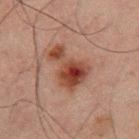Captured during whole-body skin photography for melanoma surveillance; the lesion was not biopsied. A close-up tile cropped from a whole-body skin photograph, about 15 mm across. A male subject approximately 65 years of age. The recorded lesion diameter is about 5 mm. The lesion is on the right thigh.